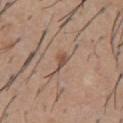Imaged during a routine full-body skin examination; the lesion was not biopsied and no histopathology is available. The lesion is located on the chest. The patient is a male roughly 60 years of age. A roughly 15 mm field-of-view crop from a total-body skin photograph.